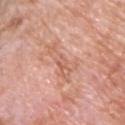No biopsy was performed on this lesion — it was imaged during a full skin examination and was not determined to be concerning. The lesion's longest dimension is about 5.5 mm. A lesion tile, about 15 mm wide, cut from a 3D total-body photograph. The lesion is located on the chest. A male subject, approximately 60 years of age. Imaged with white-light lighting.